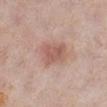biopsy status=catalogued during a skin exam; not biopsied | anatomic site=the left lower leg | acquisition=~15 mm tile from a whole-body skin photo | lighting=white-light | subject=female, aged 58 to 62 | size=≈4 mm.